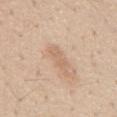{"biopsy_status": "not biopsied; imaged during a skin examination", "automated_metrics": {"eccentricity": 0.95, "shape_asymmetry": 0.2, "vs_skin_contrast_norm": 5.0, "nevus_likeness_0_100": 0}, "image": {"source": "total-body photography crop", "field_of_view_mm": 15}, "site": "chest", "patient": {"sex": "male", "age_approx": 55}}